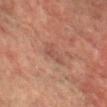Q: Was a biopsy performed?
A: catalogued during a skin exam; not biopsied
Q: What did automated image analysis measure?
A: a lesion area of about 4.5 mm² and an eccentricity of roughly 0.85; an average lesion color of about L≈41 a*≈20 b*≈23 (CIELAB), about 5 CIELAB-L* units darker than the surrounding skin, and a normalized lesion–skin contrast near 4.5
Q: What kind of image is this?
A: ~15 mm tile from a whole-body skin photo
Q: Where on the body is the lesion?
A: the back
Q: How was the tile lit?
A: cross-polarized illumination
Q: Patient demographics?
A: male, in their 70s
Q: Lesion size?
A: about 3 mm